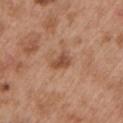biopsy status=imaged on a skin check; not biopsied | patient=male, aged 53 to 57 | tile lighting=white-light | lesion diameter=~2.5 mm (longest diameter) | imaging modality=~15 mm tile from a whole-body skin photo | site=the left upper arm.